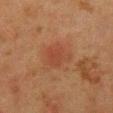notes = catalogued during a skin exam; not biopsied | acquisition = ~15 mm tile from a whole-body skin photo | patient = female, aged 48 to 52 | body site = the chest.